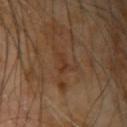<tbp_lesion>
<biopsy_status>not biopsied; imaged during a skin examination</biopsy_status>
<lighting>cross-polarized</lighting>
<site>right upper arm</site>
<lesion_size>
  <long_diameter_mm_approx>2.5</long_diameter_mm_approx>
</lesion_size>
<image>
  <source>total-body photography crop</source>
  <field_of_view_mm>15</field_of_view_mm>
</image>
<patient>
  <sex>male</sex>
  <age_approx>65</age_approx>
</patient>
</tbp_lesion>